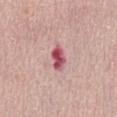The lesion was tiled from a total-body skin photograph and was not biopsied. From the front of the torso. A female patient, aged approximately 65. A 15 mm close-up extracted from a 3D total-body photography capture. The lesion-visualizer software estimated a lesion area of about 4.5 mm², an eccentricity of roughly 0.8, and two-axis asymmetry of about 0.25.